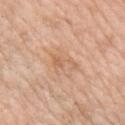Recorded during total-body skin imaging; not selected for excision or biopsy. A female subject aged 63–67. A lesion tile, about 15 mm wide, cut from a 3D total-body photograph. The lesion is located on the right upper arm.